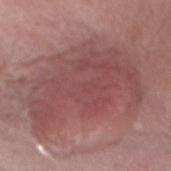Q: What kind of image is this?
A: ~15 mm tile from a whole-body skin photo
Q: Patient demographics?
A: female, aged around 65
Q: Lesion size?
A: about 11 mm
Q: What is the anatomic site?
A: the chest
Q: How was the tile lit?
A: white-light illumination
Q: Automated lesion metrics?
A: a footprint of about 65 mm², an outline eccentricity of about 0.8 (0 = round, 1 = elongated), and two-axis asymmetry of about 0.3; a classifier nevus-likeness of about 75/100 and a detector confidence of about 85 out of 100 that the crop contains a lesion